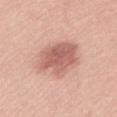  biopsy_status: not biopsied; imaged during a skin examination
  lesion_size:
    long_diameter_mm_approx: 6.0
  lighting: white-light
  image:
    source: total-body photography crop
    field_of_view_mm: 15
  patient:
    sex: female
    age_approx: 45
  automated_metrics:
    lesion_detection_confidence_0_100: 100
  site: left thigh